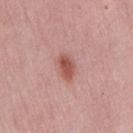workup: no biopsy performed (imaged during a skin exam)
location: the lower back
size: ~3.5 mm (longest diameter)
tile lighting: white-light illumination
patient: female, aged around 60
image: total-body-photography crop, ~15 mm field of view